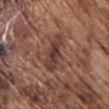Impression: The lesion was photographed on a routine skin check and not biopsied; there is no pathology result. Context: A 15 mm close-up extracted from a 3D total-body photography capture. A male subject approximately 75 years of age. Automated image analysis of the tile measured a border-irregularity index near 4/10, a color-variation rating of about 4/10, and radial color variation of about 1. The tile uses white-light illumination. The lesion is located on the chest. Longest diameter approximately 4.5 mm.